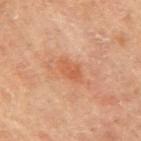The lesion was tiled from a total-body skin photograph and was not biopsied.
The lesion is located on the right upper arm.
This image is a 15 mm lesion crop taken from a total-body photograph.
The recorded lesion diameter is about 3 mm.
Captured under cross-polarized illumination.
A female patient, aged around 65.
Automated image analysis of the tile measured border irregularity of about 2.5 on a 0–10 scale, internal color variation of about 2.5 on a 0–10 scale, and a peripheral color-asymmetry measure near 1.